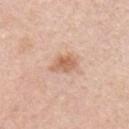Q: Is there a histopathology result?
A: imaged on a skin check; not biopsied
Q: What did automated image analysis measure?
A: a shape eccentricity near 0.7 and two-axis asymmetry of about 0.25; border irregularity of about 2.5 on a 0–10 scale and a color-variation rating of about 3.5/10
Q: Lesion location?
A: the chest
Q: How was this image acquired?
A: 15 mm crop, total-body photography
Q: Patient demographics?
A: male, approximately 45 years of age